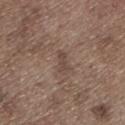Assessment: The lesion was tiled from a total-body skin photograph and was not biopsied. Image and clinical context: Automated tile analysis of the lesion measured a footprint of about 3 mm² and two-axis asymmetry of about 0.35. And it measured a border-irregularity index near 3.5/10, a within-lesion color-variation index near 1/10, and peripheral color asymmetry of about 0. The analysis additionally found an automated nevus-likeness rating near 0 out of 100 and a lesion-detection confidence of about 95/100. Longest diameter approximately 2.5 mm. On the leg. Captured under white-light illumination. Cropped from a total-body skin-imaging series; the visible field is about 15 mm. A male subject, in their 70s.